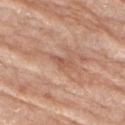workup=total-body-photography surveillance lesion; no biopsy
subject=female, aged around 75
location=the arm
imaging modality=~15 mm tile from a whole-body skin photo